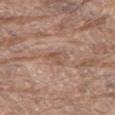The lesion was photographed on a routine skin check and not biopsied; there is no pathology result. About 3 mm across. A roughly 15 mm field-of-view crop from a total-body skin photograph. Automated image analysis of the tile measured a nevus-likeness score of about 0/100 and lesion-presence confidence of about 75/100. Captured under white-light illumination. Located on the chest. A male subject aged approximately 80.